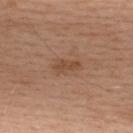This lesion was catalogued during total-body skin photography and was not selected for biopsy.
The lesion is on the upper back.
An algorithmic analysis of the crop reported a lesion area of about 4.5 mm², an eccentricity of roughly 0.9, and a shape-asymmetry score of about 0.35 (0 = symmetric). And it measured an average lesion color of about L≈48 a*≈20 b*≈31 (CIELAB), about 8 CIELAB-L* units darker than the surrounding skin, and a normalized border contrast of about 6.5. The software also gave a nevus-likeness score of about 20/100.
This is a white-light tile.
A male patient approximately 35 years of age.
About 3.5 mm across.
This image is a 15 mm lesion crop taken from a total-body photograph.